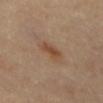Recorded during total-body skin imaging; not selected for excision or biopsy. A 15 mm close-up extracted from a 3D total-body photography capture. The patient is a female aged 48 to 52. Measured at roughly 3.5 mm in maximum diameter. From the front of the torso. The tile uses cross-polarized illumination.